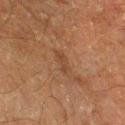Q: Was a biopsy performed?
A: catalogued during a skin exam; not biopsied
Q: What did automated image analysis measure?
A: border irregularity of about 3.5 on a 0–10 scale, a color-variation rating of about 0/10, and a peripheral color-asymmetry measure near 0; a classifier nevus-likeness of about 0/100 and a detector confidence of about 95 out of 100 that the crop contains a lesion
Q: What is the lesion's diameter?
A: ~3 mm (longest diameter)
Q: Where on the body is the lesion?
A: the right lower leg
Q: How was the tile lit?
A: cross-polarized
Q: What kind of image is this?
A: 15 mm crop, total-body photography
Q: Who is the patient?
A: male, aged approximately 60